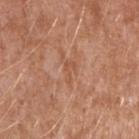Notes:
• follow-up: no biopsy performed (imaged during a skin exam)
• body site: the right upper arm
• image: ~15 mm crop, total-body skin-cancer survey
• automated lesion analysis: a footprint of about 2.5 mm², an outline eccentricity of about 0.9 (0 = round, 1 = elongated), and a symmetry-axis asymmetry near 0.7; a color-variation rating of about 0/10 and a peripheral color-asymmetry measure near 0
• diameter: about 3 mm
• subject: male, in their mid- to late 40s